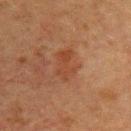Q: Is there a histopathology result?
A: imaged on a skin check; not biopsied
Q: Patient demographics?
A: female, in their mid- to late 50s
Q: How large is the lesion?
A: about 4 mm
Q: How was this image acquired?
A: 15 mm crop, total-body photography
Q: Illumination type?
A: cross-polarized
Q: Lesion location?
A: the back